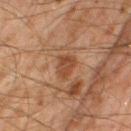Captured during whole-body skin photography for melanoma surveillance; the lesion was not biopsied. Cropped from a total-body skin-imaging series; the visible field is about 15 mm. From the right thigh. The subject is a male aged 43 to 47. Captured under cross-polarized illumination. The lesion's longest dimension is about 3 mm.